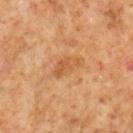<case>
<site>right lower leg</site>
<patient>
  <sex>male</sex>
  <age_approx>60</age_approx>
</patient>
<image>
  <source>total-body photography crop</source>
  <field_of_view_mm>15</field_of_view_mm>
</image>
<lesion_size>
  <long_diameter_mm_approx>4.0</long_diameter_mm_approx>
</lesion_size>
<lighting>cross-polarized</lighting>
</case>